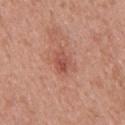| field | value |
|---|---|
| body site | the back |
| illumination | white-light illumination |
| size | ~3 mm (longest diameter) |
| patient | female, aged 48–52 |
| image | 15 mm crop, total-body photography |
| automated metrics | a lesion color around L≈52 a*≈28 b*≈29 in CIELAB, roughly 9 lightness units darker than nearby skin, and a normalized lesion–skin contrast near 6.5; a border-irregularity index near 2.5/10, internal color variation of about 4 on a 0–10 scale, and peripheral color asymmetry of about 1.5; a nevus-likeness score of about 30/100 and a lesion-detection confidence of about 100/100 |